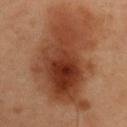Imaged during a routine full-body skin examination; the lesion was not biopsied and no histopathology is available. The subject is a male about 55 years old. The lesion is located on the right upper arm. A close-up tile cropped from a whole-body skin photograph, about 15 mm across.